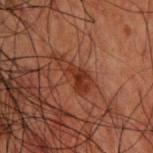Captured during whole-body skin photography for melanoma surveillance; the lesion was not biopsied. A male patient, aged around 50. Located on the upper back. The total-body-photography lesion software estimated a mean CIELAB color near L≈25 a*≈21 b*≈25 and a lesion–skin lightness drop of about 7. And it measured radial color variation of about 1. It also reported a lesion-detection confidence of about 100/100. A close-up tile cropped from a whole-body skin photograph, about 15 mm across. This is a cross-polarized tile.